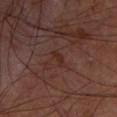* biopsy status · total-body-photography surveillance lesion; no biopsy
* subject · male, aged around 50
* image · ~15 mm tile from a whole-body skin photo
* lesion diameter · ≈2.5 mm
* location · the upper back